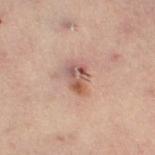{"biopsy_status": "not biopsied; imaged during a skin examination", "site": "left thigh", "lesion_size": {"long_diameter_mm_approx": 4.0}, "patient": {"sex": "male", "age_approx": 55}, "image": {"source": "total-body photography crop", "field_of_view_mm": 15}, "automated_metrics": {"shape_asymmetry": 0.2, "border_irregularity_0_10": 2.5, "color_variation_0_10": 9.0, "peripheral_color_asymmetry": 3.5, "nevus_likeness_0_100": 25, "lesion_detection_confidence_0_100": 100}}